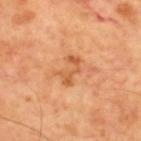• workup: imaged on a skin check; not biopsied
• site: the upper back
• subject: male, aged around 60
• TBP lesion metrics: a border-irregularity index near 7/10
• image source: ~15 mm crop, total-body skin-cancer survey
• diameter: about 3.5 mm